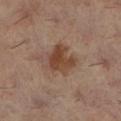biopsy_status: not biopsied; imaged during a skin examination
image:
  source: total-body photography crop
  field_of_view_mm: 15
lesion_size:
  long_diameter_mm_approx: 4.5
patient:
  sex: female
  age_approx: 60
site: left lower leg